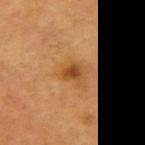Captured during whole-body skin photography for melanoma surveillance; the lesion was not biopsied. A close-up tile cropped from a whole-body skin photograph, about 15 mm across. A male subject, aged 63 to 67. The lesion is located on the right upper arm. Approximately 2 mm at its widest. Automated image analysis of the tile measured an area of roughly 3.5 mm², a shape eccentricity near 0.65, and two-axis asymmetry of about 0.25. And it measured a border-irregularity rating of about 2/10 and a color-variation rating of about 2.5/10. The software also gave a classifier nevus-likeness of about 85/100 and lesion-presence confidence of about 100/100.